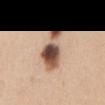follow-up: total-body-photography surveillance lesion; no biopsy | acquisition: ~15 mm tile from a whole-body skin photo | anatomic site: the chest | TBP lesion metrics: an average lesion color of about L≈53 a*≈19 b*≈28 (CIELAB), roughly 20 lightness units darker than nearby skin, and a normalized border contrast of about 13; a border-irregularity rating of about 4/10, a color-variation rating of about 7.5/10, and peripheral color asymmetry of about 1.5; a classifier nevus-likeness of about 100/100 and lesion-presence confidence of about 100/100 | size: about 6 mm | lighting: white-light | subject: female, approximately 30 years of age.